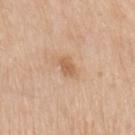Part of a total-body skin-imaging series; this lesion was reviewed on a skin check and was not flagged for biopsy.
Located on the left upper arm.
A female subject, about 65 years old.
About 2.5 mm across.
The total-body-photography lesion software estimated a footprint of about 4 mm² and a symmetry-axis asymmetry near 0.25. The software also gave a mean CIELAB color near L≈61 a*≈20 b*≈35, about 9 CIELAB-L* units darker than the surrounding skin, and a lesion-to-skin contrast of about 6.5 (normalized; higher = more distinct).
A roughly 15 mm field-of-view crop from a total-body skin photograph.
The tile uses white-light illumination.